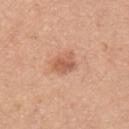Clinical impression: This lesion was catalogued during total-body skin photography and was not selected for biopsy. Clinical summary: Automated image analysis of the tile measured an area of roughly 4.5 mm² and two-axis asymmetry of about 0.2. And it measured a border-irregularity index near 1.5/10 and a color-variation rating of about 2.5/10. And it measured an automated nevus-likeness rating near 65 out of 100 and a detector confidence of about 100 out of 100 that the crop contains a lesion. A male patient roughly 45 years of age. Approximately 3 mm at its widest. A 15 mm crop from a total-body photograph taken for skin-cancer surveillance. From the arm. Captured under white-light illumination.